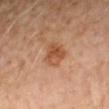Assessment: Imaged during a routine full-body skin examination; the lesion was not biopsied and no histopathology is available. Clinical summary: Approximately 3.5 mm at its widest. Cropped from a total-body skin-imaging series; the visible field is about 15 mm. The tile uses cross-polarized illumination. From the right forearm. A female subject, aged approximately 50.